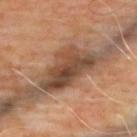Recorded during total-body skin imaging; not selected for excision or biopsy. From the upper back. Imaged with cross-polarized lighting. A male patient, approximately 65 years of age. A close-up tile cropped from a whole-body skin photograph, about 15 mm across. The recorded lesion diameter is about 7.5 mm.